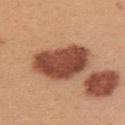Q: Is there a histopathology result?
A: total-body-photography surveillance lesion; no biopsy
Q: Illumination type?
A: white-light illumination
Q: What are the patient's age and sex?
A: female, roughly 25 years of age
Q: Lesion location?
A: the back
Q: How was this image acquired?
A: 15 mm crop, total-body photography
Q: What is the lesion's diameter?
A: ~7 mm (longest diameter)